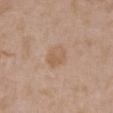<case>
<biopsy_status>not biopsied; imaged during a skin examination</biopsy_status>
<lighting>white-light</lighting>
<lesion_size>
  <long_diameter_mm_approx>3.0</long_diameter_mm_approx>
</lesion_size>
<site>right upper arm</site>
<image>
  <source>total-body photography crop</source>
  <field_of_view_mm>15</field_of_view_mm>
</image>
<patient>
  <sex>female</sex>
  <age_approx>35</age_approx>
</patient>
</case>